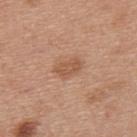follow-up: imaged on a skin check; not biopsied | body site: the upper back | subject: male, aged approximately 30 | size: about 3 mm | image: ~15 mm crop, total-body skin-cancer survey.